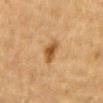| feature | finding |
|---|---|
| workup | no biopsy performed (imaged during a skin exam) |
| location | the mid back |
| diameter | ~3 mm (longest diameter) |
| tile lighting | cross-polarized illumination |
| image | ~15 mm crop, total-body skin-cancer survey |
| TBP lesion metrics | a lesion color around L≈46 a*≈19 b*≈37 in CIELAB; internal color variation of about 4 on a 0–10 scale; a classifier nevus-likeness of about 95/100 and lesion-presence confidence of about 100/100 |
| subject | male, approximately 85 years of age or older |